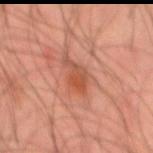Part of a total-body skin-imaging series; this lesion was reviewed on a skin check and was not flagged for biopsy.
A male patient, aged around 45.
About 4 mm across.
Cropped from a total-body skin-imaging series; the visible field is about 15 mm.
The lesion is located on the mid back.
The tile uses cross-polarized illumination.